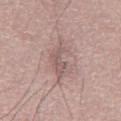tile lighting: white-light illumination
automated lesion analysis: a lesion color around L≈57 a*≈17 b*≈20 in CIELAB; a classifier nevus-likeness of about 0/100 and lesion-presence confidence of about 55/100
imaging modality: ~15 mm crop, total-body skin-cancer survey
patient: male, in their mid- to late 60s
lesion diameter: ~6.5 mm (longest diameter)
anatomic site: the abdomen